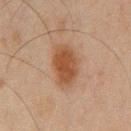Assessment: The lesion was photographed on a routine skin check and not biopsied; there is no pathology result. Image and clinical context: A male patient, approximately 45 years of age. Cropped from a total-body skin-imaging series; the visible field is about 15 mm. Imaged with cross-polarized lighting. An algorithmic analysis of the crop reported a footprint of about 13 mm² and an outline eccentricity of about 0.8 (0 = round, 1 = elongated). And it measured a within-lesion color-variation index near 3.5/10 and a peripheral color-asymmetry measure near 1. The lesion is on the chest.